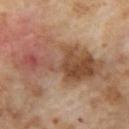No biopsy was performed on this lesion — it was imaged during a full skin examination and was not determined to be concerning. The lesion is on the left thigh. Cropped from a total-body skin-imaging series; the visible field is about 15 mm. The total-body-photography lesion software estimated a footprint of about 26 mm² and two-axis asymmetry of about 0.6. The analysis additionally found a lesion color around L≈49 a*≈20 b*≈30 in CIELAB and a lesion–skin lightness drop of about 12. The analysis additionally found a border-irregularity index near 9/10, a color-variation rating of about 7.5/10, and peripheral color asymmetry of about 2. It also reported a classifier nevus-likeness of about 0/100 and a lesion-detection confidence of about 100/100. The subject is a female roughly 55 years of age. Measured at roughly 10.5 mm in maximum diameter.